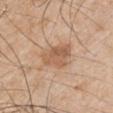* follow-up: total-body-photography surveillance lesion; no biopsy
* image source: 15 mm crop, total-body photography
* lesion diameter: ~4.5 mm (longest diameter)
* tile lighting: white-light
* site: the right upper arm
* subject: male, aged approximately 50